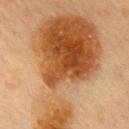Assessment: This lesion was catalogued during total-body skin photography and was not selected for biopsy. Acquisition and patient details: A female patient about 60 years old. Cropped from a whole-body photographic skin survey; the tile spans about 15 mm. Located on the front of the torso. Automated image analysis of the tile measured an area of roughly 85 mm², an outline eccentricity of about 0.9 (0 = round, 1 = elongated), and a symmetry-axis asymmetry near 0.6. And it measured an average lesion color of about L≈47 a*≈21 b*≈37 (CIELAB), a lesion–skin lightness drop of about 14, and a lesion-to-skin contrast of about 10.5 (normalized; higher = more distinct). And it measured internal color variation of about 9 on a 0–10 scale and a peripheral color-asymmetry measure near 3. And it measured an automated nevus-likeness rating near 100 out of 100. Longest diameter approximately 17 mm.